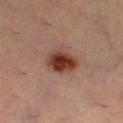Q: Was this lesion biopsied?
A: catalogued during a skin exam; not biopsied
Q: What is the imaging modality?
A: 15 mm crop, total-body photography
Q: What are the patient's age and sex?
A: female, in their mid-30s
Q: Where on the body is the lesion?
A: the left lower leg